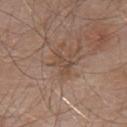Impression:
Part of a total-body skin-imaging series; this lesion was reviewed on a skin check and was not flagged for biopsy.
Acquisition and patient details:
The lesion's longest dimension is about 3 mm. The lesion is on the upper back. The subject is a male in their mid- to late 50s. Imaged with white-light lighting. This image is a 15 mm lesion crop taken from a total-body photograph. An algorithmic analysis of the crop reported a lesion area of about 4 mm², a shape eccentricity near 0.8, and two-axis asymmetry of about 0.5.